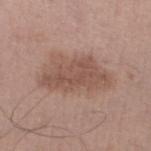Captured during whole-body skin photography for melanoma surveillance; the lesion was not biopsied.
This is a white-light tile.
Cropped from a whole-body photographic skin survey; the tile spans about 15 mm.
A male patient, roughly 50 years of age.
Located on the leg.
The total-body-photography lesion software estimated a footprint of about 27 mm², an outline eccentricity of about 0.8 (0 = round, 1 = elongated), and a shape-asymmetry score of about 0.25 (0 = symmetric). The analysis additionally found a mean CIELAB color near L≈53 a*≈19 b*≈25 and a lesion–skin lightness drop of about 9. And it measured a classifier nevus-likeness of about 15/100.
Longest diameter approximately 8 mm.